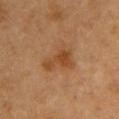notes — catalogued during a skin exam; not biopsied
acquisition — 15 mm crop, total-body photography
lesion size — about 4 mm
patient — female, approximately 60 years of age
location — the back
TBP lesion metrics — a lesion area of about 8 mm², an outline eccentricity of about 0.75 (0 = round, 1 = elongated), and a symmetry-axis asymmetry near 0.5; a mean CIELAB color near L≈40 a*≈20 b*≈33, about 7 CIELAB-L* units darker than the surrounding skin, and a lesion-to-skin contrast of about 7 (normalized; higher = more distinct); a border-irregularity rating of about 5/10, a within-lesion color-variation index near 2.5/10, and radial color variation of about 1
tile lighting — cross-polarized